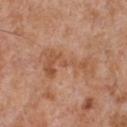automated_metrics:
  border_irregularity_0_10: 10.0
  color_variation_0_10: 3.0
  peripheral_color_asymmetry: 0.5
patient:
  sex: male
  age_approx: 65
image:
  source: total-body photography crop
  field_of_view_mm: 15
lighting: white-light
site: chest